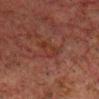Recorded during total-body skin imaging; not selected for excision or biopsy. This is a cross-polarized tile. The recorded lesion diameter is about 3.5 mm. A male subject, aged approximately 60. The lesion is located on the head or neck. A 15 mm crop from a total-body photograph taken for skin-cancer surveillance.